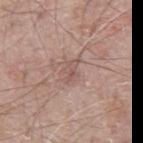Part of a total-body skin-imaging series; this lesion was reviewed on a skin check and was not flagged for biopsy.
Cropped from a total-body skin-imaging series; the visible field is about 15 mm.
Imaged with white-light lighting.
The patient is a male aged around 65.
From the abdomen.
Automated tile analysis of the lesion measured a footprint of about 4 mm² and two-axis asymmetry of about 0.35. It also reported a nevus-likeness score of about 0/100 and lesion-presence confidence of about 100/100.